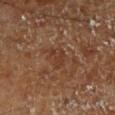  automated_metrics:
    area_mm2_approx: 5.0
    eccentricity: 0.8
    shape_asymmetry: 0.35
    cielab_L: 36
    cielab_a: 20
    cielab_b: 29
    vs_skin_darker_L: 6.0
    vs_skin_contrast_norm: 5.5
    color_variation_0_10: 2.5
  patient:
    sex: male
    age_approx: 70
  lighting: cross-polarized
  lesion_size:
    long_diameter_mm_approx: 3.5
  site: right lower leg
  image:
    source: total-body photography crop
    field_of_view_mm: 15